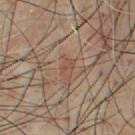This image is a 15 mm lesion crop taken from a total-body photograph.
The subject is a male aged around 55.
The lesion is located on the chest.